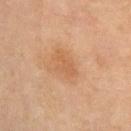| feature | finding |
|---|---|
| notes | catalogued during a skin exam; not biopsied |
| acquisition | total-body-photography crop, ~15 mm field of view |
| TBP lesion metrics | an outline eccentricity of about 0.75 (0 = round, 1 = elongated); a mean CIELAB color near L≈50 a*≈20 b*≈33, a lesion–skin lightness drop of about 6, and a normalized border contrast of about 4.5; a border-irregularity rating of about 5/10; an automated nevus-likeness rating near 75 out of 100 and a detector confidence of about 100 out of 100 that the crop contains a lesion |
| patient | female, aged 53 to 57 |
| illumination | cross-polarized illumination |
| diameter | ~3 mm (longest diameter) |
| location | the left forearm |